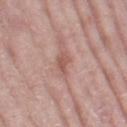Assessment: Part of a total-body skin-imaging series; this lesion was reviewed on a skin check and was not flagged for biopsy. Context: This image is a 15 mm lesion crop taken from a total-body photograph. On the left thigh. The patient is a female aged 68 to 72.